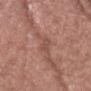  patient:
    sex: female
    age_approx: 65
  image:
    source: total-body photography crop
    field_of_view_mm: 15
  site: head or neck